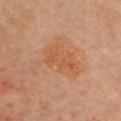Clinical impression:
The lesion was photographed on a routine skin check and not biopsied; there is no pathology result.
Clinical summary:
Imaged with cross-polarized lighting. The recorded lesion diameter is about 5 mm. From the chest. A roughly 15 mm field-of-view crop from a total-body skin photograph. The subject is a female aged around 60.